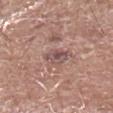lighting — white-light
site — the left lower leg
size — ~3 mm (longest diameter)
patient — male, aged approximately 65
automated metrics — a classifier nevus-likeness of about 0/100 and lesion-presence confidence of about 95/100
imaging modality — ~15 mm crop, total-body skin-cancer survey